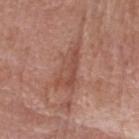<case>
<biopsy_status>not biopsied; imaged during a skin examination</biopsy_status>
<lighting>white-light</lighting>
<lesion_size>
  <long_diameter_mm_approx>5.5</long_diameter_mm_approx>
</lesion_size>
<image>
  <source>total-body photography crop</source>
  <field_of_view_mm>15</field_of_view_mm>
</image>
<automated_metrics>
  <border_irregularity_0_10>5.5</border_irregularity_0_10>
  <peripheral_color_asymmetry>1.0</peripheral_color_asymmetry>
  <nevus_likeness_0_100>0</nevus_likeness_0_100>
  <lesion_detection_confidence_0_100>50</lesion_detection_confidence_0_100>
</automated_metrics>
<site>head or neck</site>
<patient>
  <sex>male</sex>
  <age_approx>80</age_approx>
</patient>
</case>